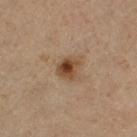Part of a total-body skin-imaging series; this lesion was reviewed on a skin check and was not flagged for biopsy.
An algorithmic analysis of the crop reported an average lesion color of about L≈45 a*≈18 b*≈32 (CIELAB), about 12 CIELAB-L* units darker than the surrounding skin, and a normalized border contrast of about 9.5. The analysis additionally found a border-irregularity rating of about 2/10, internal color variation of about 8 on a 0–10 scale, and a peripheral color-asymmetry measure near 2.5. And it measured a classifier nevus-likeness of about 95/100 and lesion-presence confidence of about 100/100.
A close-up tile cropped from a whole-body skin photograph, about 15 mm across.
A male subject aged 63–67.
From the right thigh.